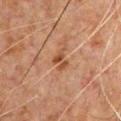{"biopsy_status": "not biopsied; imaged during a skin examination", "patient": {"sex": "male", "age_approx": 50}, "automated_metrics": {"nevus_likeness_0_100": 0, "lesion_detection_confidence_0_100": 100}, "site": "front of the torso", "image": {"source": "total-body photography crop", "field_of_view_mm": 15}, "lesion_size": {"long_diameter_mm_approx": 2.5}}